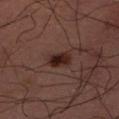Assessment:
This lesion was catalogued during total-body skin photography and was not selected for biopsy.
Background:
A lesion tile, about 15 mm wide, cut from a 3D total-body photograph. The tile uses cross-polarized illumination. The subject is a male aged approximately 60. The recorded lesion diameter is about 3 mm.